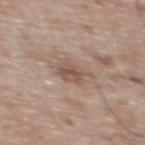This lesion was catalogued during total-body skin photography and was not selected for biopsy. Cropped from a total-body skin-imaging series; the visible field is about 15 mm. This is a white-light tile. Located on the upper back. Automated image analysis of the tile measured a footprint of about 7 mm², an eccentricity of roughly 0.6, and a shape-asymmetry score of about 0.3 (0 = symmetric). The software also gave a mean CIELAB color near L≈53 a*≈15 b*≈25, about 9 CIELAB-L* units darker than the surrounding skin, and a normalized border contrast of about 6.5. The analysis additionally found a classifier nevus-likeness of about 0/100 and a lesion-detection confidence of about 100/100. A male patient about 50 years old.